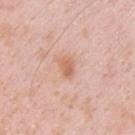The patient is a male aged 33–37. Located on the chest. This image is a 15 mm lesion crop taken from a total-body photograph.